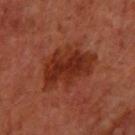<lesion>
<biopsy_status>not biopsied; imaged during a skin examination</biopsy_status>
<automated_metrics>
  <eccentricity>0.75</eccentricity>
  <shape_asymmetry>0.3</shape_asymmetry>
  <cielab_L>30</cielab_L>
  <cielab_a>28</cielab_a>
  <cielab_b>31</cielab_b>
  <vs_skin_contrast_norm>8.5</vs_skin_contrast_norm>
  <nevus_likeness_0_100>90</nevus_likeness_0_100>
  <lesion_detection_confidence_0_100>100</lesion_detection_confidence_0_100>
</automated_metrics>
<lighting>cross-polarized</lighting>
<site>left forearm</site>
<image>
  <source>total-body photography crop</source>
  <field_of_view_mm>15</field_of_view_mm>
</image>
<patient>
  <sex>female</sex>
  <age_approx>65</age_approx>
</patient>
<lesion_size>
  <long_diameter_mm_approx>7.0</long_diameter_mm_approx>
</lesion_size>
</lesion>